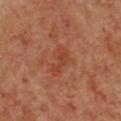{"biopsy_status": "not biopsied; imaged during a skin examination", "image": {"source": "total-body photography crop", "field_of_view_mm": 15}, "lesion_size": {"long_diameter_mm_approx": 4.0}, "patient": {"sex": "female", "age_approx": 40}, "automated_metrics": {"area_mm2_approx": 6.5, "eccentricity": 0.85, "shape_asymmetry": 0.35, "vs_skin_darker_L": 6.0, "vs_skin_contrast_norm": 5.5, "nevus_likeness_0_100": 0}, "lighting": "cross-polarized", "site": "front of the torso"}